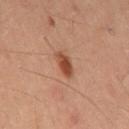Impression: Recorded during total-body skin imaging; not selected for excision or biopsy. Clinical summary: About 3 mm across. The tile uses cross-polarized illumination. The patient is a male aged around 60. On the mid back. A roughly 15 mm field-of-view crop from a total-body skin photograph.